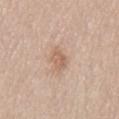Q: Is there a histopathology result?
A: imaged on a skin check; not biopsied
Q: What is the lesion's diameter?
A: ~3 mm (longest diameter)
Q: Where on the body is the lesion?
A: the lower back
Q: Illumination type?
A: white-light
Q: Automated lesion metrics?
A: a footprint of about 4.5 mm², a shape eccentricity near 0.8, and a symmetry-axis asymmetry near 0.3; an automated nevus-likeness rating near 5 out of 100 and a lesion-detection confidence of about 100/100
Q: Who is the patient?
A: male, aged approximately 75
Q: What is the imaging modality?
A: ~15 mm crop, total-body skin-cancer survey